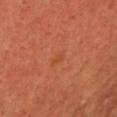Recorded during total-body skin imaging; not selected for excision or biopsy. A female patient in their 50s. A 15 mm close-up extracted from a 3D total-body photography capture. The lesion's longest dimension is about 2.5 mm. Automated tile analysis of the lesion measured a footprint of about 2.5 mm², an outline eccentricity of about 0.85 (0 = round, 1 = elongated), and a symmetry-axis asymmetry near 0.25.